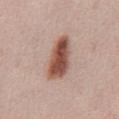follow-up — imaged on a skin check; not biopsied
anatomic site — the abdomen
illumination — white-light illumination
subject — male, approximately 50 years of age
lesion size — ≈5.5 mm
image — ~15 mm tile from a whole-body skin photo